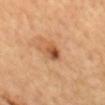Imaged during a routine full-body skin examination; the lesion was not biopsied and no histopathology is available. The lesion's longest dimension is about 2.5 mm. From the mid back. The total-body-photography lesion software estimated an eccentricity of roughly 0.65 and a shape-asymmetry score of about 0.2 (0 = symmetric). A lesion tile, about 15 mm wide, cut from a 3D total-body photograph. A male patient roughly 65 years of age. This is a cross-polarized tile.